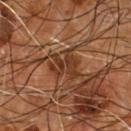notes: imaged on a skin check; not biopsied
subject: male, roughly 55 years of age
imaging modality: 15 mm crop, total-body photography
body site: the chest